The lesion is on the left lower leg. A 15 mm crop from a total-body photograph taken for skin-cancer surveillance. An algorithmic analysis of the crop reported a border-irregularity rating of about 1.5/10 and a color-variation rating of about 6/10. The subject is a female aged 38–42. Imaged with white-light lighting. Longest diameter approximately 6 mm.
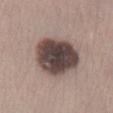{"diagnosis": {"histopathology": "dysplastic (Clark) nevus", "malignancy": "benign", "taxonomic_path": ["Benign", "Benign melanocytic proliferations", "Nevus", "Nevus, Atypical, Dysplastic, or Clark"]}}